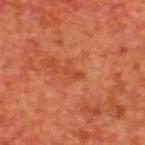Captured during whole-body skin photography for melanoma surveillance; the lesion was not biopsied. A male subject aged 63–67. A 15 mm close-up tile from a total-body photography series done for melanoma screening. The lesion is on the upper back. The tile uses cross-polarized illumination.